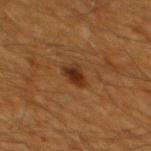  biopsy_status: not biopsied; imaged during a skin examination
  patient:
    sex: male
    age_approx: 60
  image:
    source: total-body photography crop
    field_of_view_mm: 15
  site: mid back
  lesion_size:
    long_diameter_mm_approx: 2.5
  lighting: cross-polarized